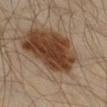{
  "patient": {
    "sex": "male",
    "age_approx": 45
  },
  "image": {
    "source": "total-body photography crop",
    "field_of_view_mm": 15
  },
  "site": "leg",
  "lesion_size": {
    "long_diameter_mm_approx": 3.5
  }
}